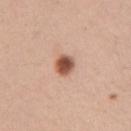notes = catalogued during a skin exam; not biopsied
patient = female, in their 30s
acquisition = ~15 mm tile from a whole-body skin photo
body site = the chest